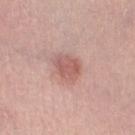Imaged during a routine full-body skin examination; the lesion was not biopsied and no histopathology is available.
Located on the left thigh.
The recorded lesion diameter is about 3 mm.
Automated tile analysis of the lesion measured a lesion area of about 6.5 mm², an eccentricity of roughly 0.35, and a shape-asymmetry score of about 0.25 (0 = symmetric). It also reported an average lesion color of about L≈58 a*≈24 b*≈24 (CIELAB), a lesion–skin lightness drop of about 10, and a normalized lesion–skin contrast near 6.5. It also reported a classifier nevus-likeness of about 70/100 and lesion-presence confidence of about 100/100.
Imaged with white-light lighting.
The subject is a female roughly 65 years of age.
This image is a 15 mm lesion crop taken from a total-body photograph.